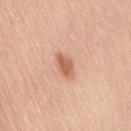Assessment:
The lesion was tiled from a total-body skin photograph and was not biopsied.
Clinical summary:
The lesion is on the back. Cropped from a total-body skin-imaging series; the visible field is about 15 mm. Approximately 3.5 mm at its widest. The lesion-visualizer software estimated a lesion color around L≈62 a*≈23 b*≈34 in CIELAB, a lesion–skin lightness drop of about 11, and a lesion-to-skin contrast of about 7.5 (normalized; higher = more distinct). It also reported a color-variation rating of about 2.5/10 and radial color variation of about 1. The software also gave a classifier nevus-likeness of about 95/100. A female patient aged around 65.